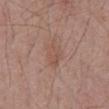| feature | finding |
|---|---|
| site | the abdomen |
| subject | male, in their 70s |
| tile lighting | white-light illumination |
| image source | ~15 mm crop, total-body skin-cancer survey |